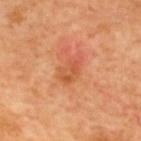Recorded during total-body skin imaging; not selected for excision or biopsy.
The tile uses cross-polarized illumination.
A close-up tile cropped from a whole-body skin photograph, about 15 mm across.
From the upper back.
A male patient aged 58 to 62.